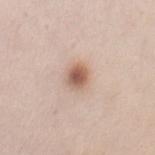Findings:
– biopsy status · imaged on a skin check; not biopsied
– lighting · white-light
– subject · female, approximately 45 years of age
– automated lesion analysis · an area of roughly 5 mm², an eccentricity of roughly 0.6, and a shape-asymmetry score of about 0.15 (0 = symmetric); border irregularity of about 1.5 on a 0–10 scale, internal color variation of about 5 on a 0–10 scale, and peripheral color asymmetry of about 1.5; a nevus-likeness score of about 95/100 and a detector confidence of about 100 out of 100 that the crop contains a lesion
– anatomic site · the chest
– imaging modality · 15 mm crop, total-body photography
– lesion size · about 2.5 mm